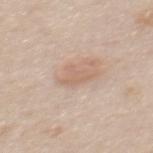follow-up: catalogued during a skin exam; not biopsied | location: the mid back | subject: female, aged 38 to 42 | imaging modality: ~15 mm tile from a whole-body skin photo.